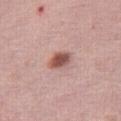biopsy status = no biopsy performed (imaged during a skin exam)
diameter = ≈3 mm
imaging modality = ~15 mm crop, total-body skin-cancer survey
subject = female, about 50 years old
TBP lesion metrics = a lesion color around L≈54 a*≈23 b*≈25 in CIELAB, a lesion–skin lightness drop of about 14, and a normalized border contrast of about 9.5; border irregularity of about 1.5 on a 0–10 scale, a within-lesion color-variation index near 4/10, and a peripheral color-asymmetry measure near 1.5
site = the right thigh
lighting = white-light illumination